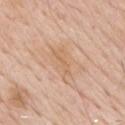On the chest. A lesion tile, about 15 mm wide, cut from a 3D total-body photograph. A male subject aged around 65.